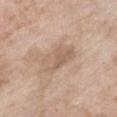Captured during whole-body skin photography for melanoma surveillance; the lesion was not biopsied. Located on the chest. Imaged with white-light lighting. About 5 mm across. A female subject approximately 75 years of age. A 15 mm close-up tile from a total-body photography series done for melanoma screening.